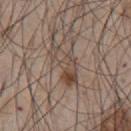The lesion was photographed on a routine skin check and not biopsied; there is no pathology result.
On the front of the torso.
A close-up tile cropped from a whole-body skin photograph, about 15 mm across.
The recorded lesion diameter is about 5 mm.
The total-body-photography lesion software estimated a lesion area of about 9.5 mm², a shape eccentricity near 0.9, and two-axis asymmetry of about 0.45. And it measured an average lesion color of about L≈47 a*≈14 b*≈24 (CIELAB), about 8 CIELAB-L* units darker than the surrounding skin, and a lesion-to-skin contrast of about 6.5 (normalized; higher = more distinct). The software also gave a border-irregularity rating of about 5.5/10 and a within-lesion color-variation index near 9/10. And it measured an automated nevus-likeness rating near 0 out of 100 and a detector confidence of about 95 out of 100 that the crop contains a lesion.
A male patient about 45 years old.
The tile uses white-light illumination.